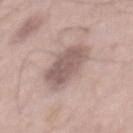No biopsy was performed on this lesion — it was imaged during a full skin examination and was not determined to be concerning. A male patient roughly 55 years of age. From the mid back. A 15 mm close-up tile from a total-body photography series done for melanoma screening.